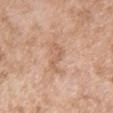Captured during whole-body skin photography for melanoma surveillance; the lesion was not biopsied.